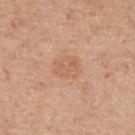follow-up: catalogued during a skin exam; not biopsied
anatomic site: the arm
imaging modality: total-body-photography crop, ~15 mm field of view
subject: female, aged approximately 40
diameter: about 3 mm
automated metrics: an area of roughly 6 mm² and an outline eccentricity of about 0.6 (0 = round, 1 = elongated); a color-variation rating of about 2.5/10 and peripheral color asymmetry of about 1; an automated nevus-likeness rating near 5 out of 100